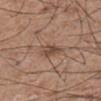This image is a 15 mm lesion crop taken from a total-body photograph. Located on the front of the torso. A male subject, in their mid- to late 50s.